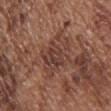anatomic site = the chest
imaging modality = total-body-photography crop, ~15 mm field of view
size = about 5 mm
automated lesion analysis = an average lesion color of about L≈41 a*≈21 b*≈25 (CIELAB), a lesion–skin lightness drop of about 7, and a normalized border contrast of about 6.5; a detector confidence of about 90 out of 100 that the crop contains a lesion
lighting = white-light
subject = male, aged approximately 75